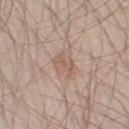No biopsy was performed on this lesion — it was imaged during a full skin examination and was not determined to be concerning. The patient is a male roughly 35 years of age. The lesion is located on the leg. A 15 mm crop from a total-body photograph taken for skin-cancer surveillance.